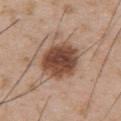Imaged during a routine full-body skin examination; the lesion was not biopsied and no histopathology is available. A male patient, approximately 55 years of age. The lesion is located on the upper back. About 5 mm across. Automated tile analysis of the lesion measured a mean CIELAB color near L≈46 a*≈20 b*≈28, a lesion–skin lightness drop of about 16, and a normalized border contrast of about 11.5. And it measured a nevus-likeness score of about 80/100 and a detector confidence of about 100 out of 100 that the crop contains a lesion. A roughly 15 mm field-of-view crop from a total-body skin photograph. This is a white-light tile.